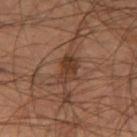The lesion was tiled from a total-body skin photograph and was not biopsied.
An algorithmic analysis of the crop reported border irregularity of about 3 on a 0–10 scale.
The lesion's longest dimension is about 3.5 mm.
Captured under cross-polarized illumination.
A 15 mm close-up tile from a total-body photography series done for melanoma screening.
On the leg.
A male patient, roughly 55 years of age.